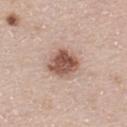follow-up = total-body-photography surveillance lesion; no biopsy
body site = the back
image-analysis metrics = a lesion–skin lightness drop of about 16 and a normalized border contrast of about 10.5; a border-irregularity rating of about 1.5/10, internal color variation of about 5 on a 0–10 scale, and peripheral color asymmetry of about 2
illumination = white-light
lesion diameter = ~3.5 mm (longest diameter)
patient = male, about 40 years old
acquisition = 15 mm crop, total-body photography